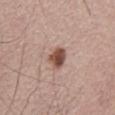biopsy_status: not biopsied; imaged during a skin examination
lighting: white-light
image:
  source: total-body photography crop
  field_of_view_mm: 15
automated_metrics:
  border_irregularity_0_10: 2.0
  color_variation_0_10: 4.0
patient:
  sex: male
  age_approx: 55